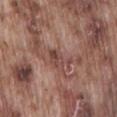lesion diameter: about 3.5 mm; subject: male, about 75 years old; imaging modality: ~15 mm crop, total-body skin-cancer survey; illumination: white-light; body site: the lower back.